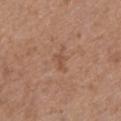automated lesion analysis: a footprint of about 4 mm² and two-axis asymmetry of about 0.45; a mean CIELAB color near L≈52 a*≈20 b*≈30 and a lesion-to-skin contrast of about 4.5 (normalized; higher = more distinct) | lesion diameter: about 3.5 mm | site: the chest | subject: female, aged 63–67 | tile lighting: white-light | image source: ~15 mm tile from a whole-body skin photo.